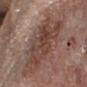{"biopsy_status": "not biopsied; imaged during a skin examination", "site": "right forearm", "image": {"source": "total-body photography crop", "field_of_view_mm": 15}, "patient": {"sex": "male", "age_approx": "85+"}, "lesion_size": {"long_diameter_mm_approx": 7.5}, "automated_metrics": {"cielab_L": 40, "cielab_a": 17, "cielab_b": 23, "vs_skin_darker_L": 8.0}, "lighting": "cross-polarized"}